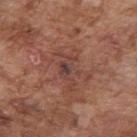Imaged during a routine full-body skin examination; the lesion was not biopsied and no histopathology is available. Located on the right upper arm. Captured under white-light illumination. The lesion's longest dimension is about 5 mm. An algorithmic analysis of the crop reported a lesion area of about 7.5 mm² and a shape eccentricity near 0.85. And it measured a lesion color around L≈42 a*≈22 b*≈24 in CIELAB and a lesion–skin lightness drop of about 7. Cropped from a total-body skin-imaging series; the visible field is about 15 mm. The patient is a male aged approximately 75.